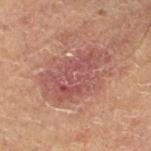{
  "biopsy_status": "not biopsied; imaged during a skin examination",
  "site": "left thigh",
  "image": {
    "source": "total-body photography crop",
    "field_of_view_mm": 15
  },
  "patient": {
    "sex": "male",
    "age_approx": 65
  },
  "automated_metrics": {
    "area_mm2_approx": 24.0,
    "eccentricity": 0.8,
    "cielab_L": 46,
    "cielab_a": 23,
    "cielab_b": 22,
    "vs_skin_darker_L": 8.0,
    "vs_skin_contrast_norm": 7.0,
    "border_irregularity_0_10": 6.0,
    "color_variation_0_10": 4.0,
    "lesion_detection_confidence_0_100": 100
  },
  "lesion_size": {
    "long_diameter_mm_approx": 7.5
  }
}